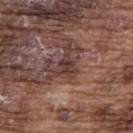Impression: No biopsy was performed on this lesion — it was imaged during a full skin examination and was not determined to be concerning. Background: Automated image analysis of the tile measured an area of roughly 4 mm² and a shape eccentricity near 0.5. The software also gave a lesion–skin lightness drop of about 9 and a normalized border contrast of about 8. And it measured a classifier nevus-likeness of about 0/100 and a lesion-detection confidence of about 85/100. Cropped from a whole-body photographic skin survey; the tile spans about 15 mm. Longest diameter approximately 2.5 mm. This is a white-light tile. A male patient roughly 75 years of age. Located on the upper back.